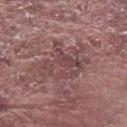workup — catalogued during a skin exam; not biopsied
TBP lesion metrics — a lesion area of about 18 mm², an outline eccentricity of about 0.75 (0 = round, 1 = elongated), and a shape-asymmetry score of about 0.35 (0 = symmetric); roughly 8 lightness units darker than nearby skin; a border-irregularity index near 7/10, a color-variation rating of about 4.5/10, and peripheral color asymmetry of about 1.5; a nevus-likeness score of about 0/100 and a detector confidence of about 65 out of 100 that the crop contains a lesion
image source — 15 mm crop, total-body photography
site — the arm
subject — male, roughly 65 years of age